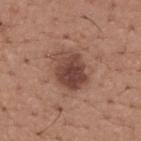{"biopsy_status": "not biopsied; imaged during a skin examination", "patient": {"sex": "male", "age_approx": 65}, "lesion_size": {"long_diameter_mm_approx": 4.5}, "image": {"source": "total-body photography crop", "field_of_view_mm": 15}, "site": "upper back"}